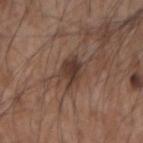Captured during whole-body skin photography for melanoma surveillance; the lesion was not biopsied.
The recorded lesion diameter is about 3 mm.
On the left forearm.
The total-body-photography lesion software estimated a mean CIELAB color near L≈37 a*≈17 b*≈23 and roughly 10 lightness units darker than nearby skin.
A male patient approximately 55 years of age.
A roughly 15 mm field-of-view crop from a total-body skin photograph.